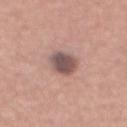  automated_metrics:
    cielab_L: 52
    cielab_a: 18
    cielab_b: 20
    vs_skin_darker_L: 15.0
    vs_skin_contrast_norm: 10.5
    nevus_likeness_0_100: 65
    lesion_detection_confidence_0_100: 100
  patient:
    sex: male
    age_approx: 45
  image:
    source: total-body photography crop
    field_of_view_mm: 15
  lighting: white-light
  lesion_size:
    long_diameter_mm_approx: 4.0
  site: mid back